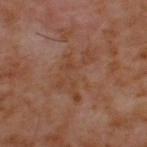  biopsy_status: not biopsied; imaged during a skin examination